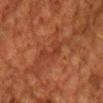The lesion was tiled from a total-body skin photograph and was not biopsied. The lesion is on the chest. Cropped from a whole-body photographic skin survey; the tile spans about 15 mm. The subject is a male aged around 60.